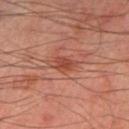Case summary:
– follow-up: total-body-photography surveillance lesion; no biopsy
– lesion size: ~2.5 mm (longest diameter)
– anatomic site: the right thigh
– patient: male, in their mid- to late 60s
– automated metrics: an eccentricity of roughly 0.75 and a shape-asymmetry score of about 0.35 (0 = symmetric); a nevus-likeness score of about 30/100 and a detector confidence of about 100 out of 100 that the crop contains a lesion
– illumination: cross-polarized
– acquisition: 15 mm crop, total-body photography